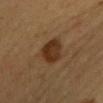<case>
<biopsy_status>not biopsied; imaged during a skin examination</biopsy_status>
<image>
  <source>total-body photography crop</source>
  <field_of_view_mm>15</field_of_view_mm>
</image>
<patient>
  <sex>female</sex>
  <age_approx>30</age_approx>
</patient>
<site>left upper arm</site>
</case>